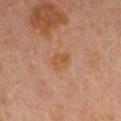Notes:
• workup — no biopsy performed (imaged during a skin exam)
• location — the left upper arm
• subject — female, about 50 years old
• image source — 15 mm crop, total-body photography
• automated lesion analysis — a footprint of about 5 mm² and a shape eccentricity near 0.5; a classifier nevus-likeness of about 25/100
• illumination — cross-polarized